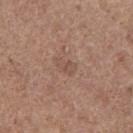Recorded during total-body skin imaging; not selected for excision or biopsy. Located on the leg. The patient is a female aged approximately 65. The tile uses white-light illumination. Cropped from a total-body skin-imaging series; the visible field is about 15 mm.